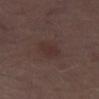– notes — total-body-photography surveillance lesion; no biopsy
– subject — male, aged approximately 70
– lesion size — about 2.5 mm
– acquisition — 15 mm crop, total-body photography
– location — the right thigh